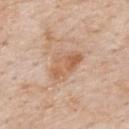biopsy status — imaged on a skin check; not biopsied
imaging modality — ~15 mm crop, total-body skin-cancer survey
patient — male, in their mid-80s or older
body site — the back